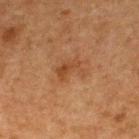| key | value |
|---|---|
| workup | imaged on a skin check; not biopsied |
| site | the upper back |
| image | ~15 mm crop, total-body skin-cancer survey |
| subject | male, in their mid- to late 60s |
| tile lighting | cross-polarized |